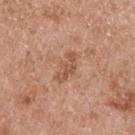Impression: Imaged during a routine full-body skin examination; the lesion was not biopsied and no histopathology is available. Image and clinical context: The lesion is on the upper back. Automated tile analysis of the lesion measured a footprint of about 5.5 mm², an outline eccentricity of about 0.9 (0 = round, 1 = elongated), and two-axis asymmetry of about 0.35. It also reported a border-irregularity index near 4/10 and a peripheral color-asymmetry measure near 0.5. The software also gave an automated nevus-likeness rating near 0 out of 100 and lesion-presence confidence of about 100/100. Measured at roughly 4 mm in maximum diameter. Imaged with white-light lighting. A male patient in their mid-50s. A close-up tile cropped from a whole-body skin photograph, about 15 mm across.